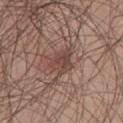This lesion was catalogued during total-body skin photography and was not selected for biopsy. From the right thigh. A male patient aged 53–57. Cropped from a total-body skin-imaging series; the visible field is about 15 mm. The lesion-visualizer software estimated about 8 CIELAB-L* units darker than the surrounding skin and a normalized border contrast of about 6.5. The software also gave an automated nevus-likeness rating near 30 out of 100 and a detector confidence of about 95 out of 100 that the crop contains a lesion. The lesion's longest dimension is about 3.5 mm. Captured under white-light illumination.